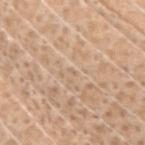Clinical impression:
Recorded during total-body skin imaging; not selected for excision or biopsy.
Context:
This image is a 15 mm lesion crop taken from a total-body photograph. The lesion is located on the arm. Captured under white-light illumination. The lesion's longest dimension is about 1 mm. A male patient, aged approximately 40. An algorithmic analysis of the crop reported an area of roughly 1 mm², a shape eccentricity near 0.8, and a symmetry-axis asymmetry near 0.5. The software also gave an average lesion color of about L≈65 a*≈16 b*≈32 (CIELAB), roughly 4 lightness units darker than nearby skin, and a normalized border contrast of about 3. It also reported border irregularity of about 3.5 on a 0–10 scale, a color-variation rating of about 0/10, and a peripheral color-asymmetry measure near 0. It also reported a nevus-likeness score of about 0/100 and a lesion-detection confidence of about 30/100.